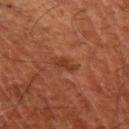Q: Was this lesion biopsied?
A: total-body-photography surveillance lesion; no biopsy
Q: Who is the patient?
A: male, aged 48 to 52
Q: What is the anatomic site?
A: the left upper arm
Q: What lighting was used for the tile?
A: cross-polarized
Q: Lesion size?
A: ~3 mm (longest diameter)
Q: What is the imaging modality?
A: total-body-photography crop, ~15 mm field of view